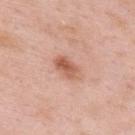workup: no biopsy performed (imaged during a skin exam)
size: ~3.5 mm (longest diameter)
image source: ~15 mm tile from a whole-body skin photo
patient: male, roughly 55 years of age
site: the upper back
tile lighting: white-light illumination